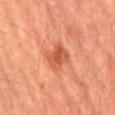Q: Is there a histopathology result?
A: no biopsy performed (imaged during a skin exam)
Q: Where on the body is the lesion?
A: the abdomen
Q: What kind of image is this?
A: ~15 mm tile from a whole-body skin photo
Q: Patient demographics?
A: male, about 65 years old
Q: How large is the lesion?
A: ≈3.5 mm
Q: How was the tile lit?
A: cross-polarized